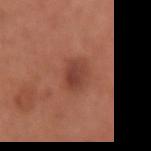The lesion was photographed on a routine skin check and not biopsied; there is no pathology result. Cropped from a total-body skin-imaging series; the visible field is about 15 mm. Automated tile analysis of the lesion measured an area of roughly 5.5 mm² and two-axis asymmetry of about 0.15. And it measured a detector confidence of about 100 out of 100 that the crop contains a lesion. A female patient, in their mid-60s. The recorded lesion diameter is about 3 mm. Located on the right forearm.